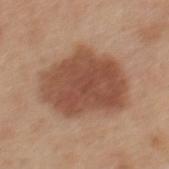follow-up: total-body-photography surveillance lesion; no biopsy
lesion size: about 8 mm
lighting: white-light illumination
automated metrics: a border-irregularity index near 2/10, internal color variation of about 4 on a 0–10 scale, and peripheral color asymmetry of about 1
image: 15 mm crop, total-body photography
patient: male, about 30 years old
location: the mid back